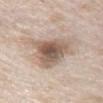Notes:
- TBP lesion metrics — a mean CIELAB color near L≈58 a*≈14 b*≈26 and about 14 CIELAB-L* units darker than the surrounding skin; a classifier nevus-likeness of about 15/100 and lesion-presence confidence of about 100/100
- image source — ~15 mm crop, total-body skin-cancer survey
- anatomic site — the chest
- patient — female, aged approximately 65
- size — about 6.5 mm
- tile lighting — white-light illumination